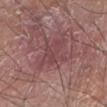Part of a total-body skin-imaging series; this lesion was reviewed on a skin check and was not flagged for biopsy. On the left lower leg. A male subject roughly 60 years of age. The lesion-visualizer software estimated an area of roughly 10 mm², an outline eccentricity of about 0.5 (0 = round, 1 = elongated), and a symmetry-axis asymmetry near 0.35. The software also gave a lesion color around L≈44 a*≈23 b*≈18 in CIELAB, a lesion–skin lightness drop of about 6, and a normalized border contrast of about 4.5. Approximately 4.5 mm at its widest. A 15 mm close-up extracted from a 3D total-body photography capture.